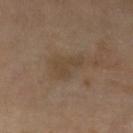Clinical impression: Imaged during a routine full-body skin examination; the lesion was not biopsied and no histopathology is available. Image and clinical context: The patient is a female roughly 70 years of age. Automated image analysis of the tile measured a mean CIELAB color near L≈43 a*≈13 b*≈28, roughly 6 lightness units darker than nearby skin, and a normalized border contrast of about 5.5. The analysis additionally found border irregularity of about 4 on a 0–10 scale. It also reported an automated nevus-likeness rating near 0 out of 100 and a detector confidence of about 100 out of 100 that the crop contains a lesion. Imaged with cross-polarized lighting. A region of skin cropped from a whole-body photographic capture, roughly 15 mm wide. On the left lower leg. The lesion's longest dimension is about 4 mm.